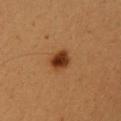Part of a total-body skin-imaging series; this lesion was reviewed on a skin check and was not flagged for biopsy. A 15 mm close-up tile from a total-body photography series done for melanoma screening. A female patient in their mid- to late 20s. The lesion is located on the head or neck.